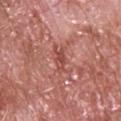biopsy_status: not biopsied; imaged during a skin examination
lighting: white-light
patient:
  sex: male
  age_approx: 65
site: upper back
lesion_size:
  long_diameter_mm_approx: 3.0
image:
  source: total-body photography crop
  field_of_view_mm: 15